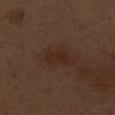Q: Was a biopsy performed?
A: imaged on a skin check; not biopsied
Q: What are the patient's age and sex?
A: male, aged 68–72
Q: Lesion size?
A: ≈3.5 mm
Q: Lesion location?
A: the right thigh
Q: What kind of image is this?
A: ~15 mm crop, total-body skin-cancer survey
Q: What lighting was used for the tile?
A: white-light illumination
Q: What did automated image analysis measure?
A: a footprint of about 5.5 mm², a shape eccentricity near 0.85, and two-axis asymmetry of about 0.25